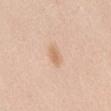This lesion was catalogued during total-body skin photography and was not selected for biopsy. The lesion is located on the front of the torso. Approximately 2.5 mm at its widest. The patient is a female aged 38–42. Captured under white-light illumination. A 15 mm close-up tile from a total-body photography series done for melanoma screening.